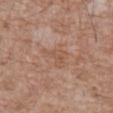Findings:
- follow-up — no biopsy performed (imaged during a skin exam)
- subject — male, roughly 60 years of age
- illumination — white-light
- size — about 3.5 mm
- site — the back
- imaging modality — total-body-photography crop, ~15 mm field of view